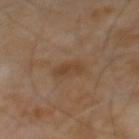follow-up — no biopsy performed (imaged during a skin exam) | subject — male, roughly 65 years of age | image — ~15 mm crop, total-body skin-cancer survey.